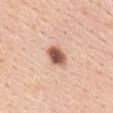Findings:
• workup · total-body-photography surveillance lesion; no biopsy
• lighting · white-light illumination
• lesion diameter · ~3.5 mm (longest diameter)
• image source · 15 mm crop, total-body photography
• automated lesion analysis · a border-irregularity rating of about 1.5/10 and peripheral color asymmetry of about 1
• location · the back
• subject · female, aged approximately 45